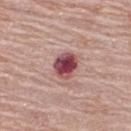notes = imaged on a skin check; not biopsied | patient = female, about 65 years old | body site = the upper back | imaging modality = ~15 mm tile from a whole-body skin photo.